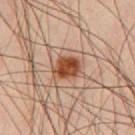workup: total-body-photography surveillance lesion; no biopsy | illumination: cross-polarized | automated lesion analysis: a peripheral color-asymmetry measure near 1.5; a classifier nevus-likeness of about 100/100 and lesion-presence confidence of about 100/100 | subject: male, in their mid- to late 40s | anatomic site: the chest | image: ~15 mm crop, total-body skin-cancer survey.